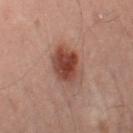follow-up = catalogued during a skin exam; not biopsied | illumination = cross-polarized illumination | subject = male, about 50 years old | location = the left lower leg | TBP lesion metrics = a normalized lesion–skin contrast near 9.5; border irregularity of about 1.5 on a 0–10 scale, a color-variation rating of about 4.5/10, and peripheral color asymmetry of about 1; a nevus-likeness score of about 100/100 and a lesion-detection confidence of about 100/100 | image source = 15 mm crop, total-body photography.